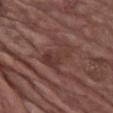Q: Illumination type?
A: white-light illumination
Q: Automated lesion metrics?
A: a lesion-detection confidence of about 55/100
Q: Who is the patient?
A: male, aged 83 or older
Q: How was this image acquired?
A: ~15 mm crop, total-body skin-cancer survey
Q: What is the lesion's diameter?
A: ~4 mm (longest diameter)
Q: Where on the body is the lesion?
A: the chest